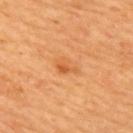A close-up tile cropped from a whole-body skin photograph, about 15 mm across. The patient is a female aged around 45. Measured at roughly 3 mm in maximum diameter. From the back. Captured under cross-polarized illumination.